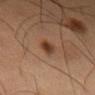biopsy_status: not biopsied; imaged during a skin examination
lesion_size:
  long_diameter_mm_approx: 3.0
lighting: cross-polarized
image:
  source: total-body photography crop
  field_of_view_mm: 15
patient:
  sex: male
  age_approx: 60
site: left thigh